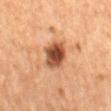Q: What are the patient's age and sex?
A: female, aged around 60
Q: How was this image acquired?
A: 15 mm crop, total-body photography
Q: How large is the lesion?
A: about 3.5 mm
Q: What is the anatomic site?
A: the mid back
Q: Illumination type?
A: cross-polarized illumination